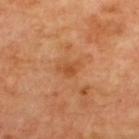The lesion was photographed on a routine skin check and not biopsied; there is no pathology result. About 2.5 mm across. A 15 mm close-up extracted from a 3D total-body photography capture. The patient is aged around 65. From the upper back.